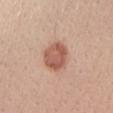Recorded during total-body skin imaging; not selected for excision or biopsy. A female subject, in their mid-20s. A 15 mm close-up extracted from a 3D total-body photography capture. Located on the left forearm.